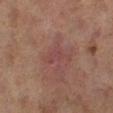{"biopsy_status": "not biopsied; imaged during a skin examination", "image": {"source": "total-body photography crop", "field_of_view_mm": 15}, "automated_metrics": {"nevus_likeness_0_100": 0, "lesion_detection_confidence_0_100": 100}, "lesion_size": {"long_diameter_mm_approx": 3.5}, "patient": {"sex": "female", "age_approx": 60}, "lighting": "cross-polarized", "site": "leg"}